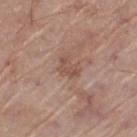Assessment:
The lesion was tiled from a total-body skin photograph and was not biopsied.
Clinical summary:
The lesion is located on the left thigh. Cropped from a whole-body photographic skin survey; the tile spans about 15 mm. The patient is a male about 70 years old.